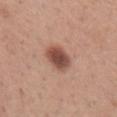Imaged during a routine full-body skin examination; the lesion was not biopsied and no histopathology is available. Captured under white-light illumination. Approximately 3.5 mm at its widest. A roughly 15 mm field-of-view crop from a total-body skin photograph. An algorithmic analysis of the crop reported a lesion color around L≈48 a*≈23 b*≈26 in CIELAB, roughly 15 lightness units darker than nearby skin, and a normalized lesion–skin contrast near 10.5. And it measured a border-irregularity rating of about 2/10, a color-variation rating of about 4/10, and peripheral color asymmetry of about 1. The lesion is located on the left forearm. A female patient, aged approximately 30.